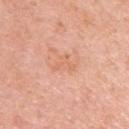follow-up: total-body-photography surveillance lesion; no biopsy
patient: female, aged around 40
image: ~15 mm tile from a whole-body skin photo
lesion size: ~3 mm (longest diameter)
automated metrics: a mean CIELAB color near L≈66 a*≈25 b*≈35, a lesion–skin lightness drop of about 6, and a normalized lesion–skin contrast near 4.5; a border-irregularity rating of about 7.5/10 and a within-lesion color-variation index near 0/10
body site: the left upper arm
illumination: white-light